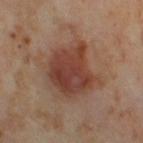subject: female, aged approximately 55 | lesion size: ≈6 mm | tile lighting: cross-polarized illumination | TBP lesion metrics: a lesion color around L≈41 a*≈22 b*≈27 in CIELAB, roughly 11 lightness units darker than nearby skin, and a lesion-to-skin contrast of about 9 (normalized; higher = more distinct); a border-irregularity index near 3/10, a color-variation rating of about 5/10, and a peripheral color-asymmetry measure near 1.5; an automated nevus-likeness rating near 100 out of 100 and lesion-presence confidence of about 100/100 | body site: the leg | image: ~15 mm tile from a whole-body skin photo.